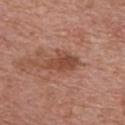Impression:
The lesion was tiled from a total-body skin photograph and was not biopsied.
Image and clinical context:
This is a white-light tile. The patient is a male roughly 70 years of age. The lesion is located on the front of the torso. Automated image analysis of the tile measured a border-irregularity index near 2.5/10, a color-variation rating of about 2.5/10, and peripheral color asymmetry of about 1. And it measured a classifier nevus-likeness of about 10/100. Cropped from a total-body skin-imaging series; the visible field is about 15 mm. The recorded lesion diameter is about 4 mm.